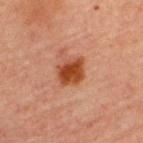anatomic site: the upper back
imaging modality: total-body-photography crop, ~15 mm field of view
size: ~3 mm (longest diameter)
subject: male, aged approximately 60
tile lighting: cross-polarized